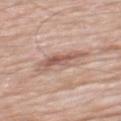Case summary:
– workup: no biopsy performed (imaged during a skin exam)
– location: the mid back
– subject: male, about 80 years old
– imaging modality: total-body-photography crop, ~15 mm field of view
– size: about 5 mm
– tile lighting: white-light illumination